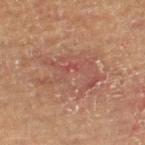{
  "site": "left thigh",
  "patient": {
    "sex": "male",
    "age_approx": 65
  },
  "lesion_size": {
    "long_diameter_mm_approx": 7.5
  },
  "image": {
    "source": "total-body photography crop",
    "field_of_view_mm": 15
  }
}